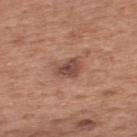Q: Was a biopsy performed?
A: no biopsy performed (imaged during a skin exam)
Q: What lighting was used for the tile?
A: white-light
Q: What did automated image analysis measure?
A: a mean CIELAB color near L≈48 a*≈22 b*≈26, roughly 11 lightness units darker than nearby skin, and a normalized lesion–skin contrast near 8; border irregularity of about 2 on a 0–10 scale, a within-lesion color-variation index near 3/10, and peripheral color asymmetry of about 1
Q: How large is the lesion?
A: about 3 mm
Q: Who is the patient?
A: male, aged approximately 55
Q: What kind of image is this?
A: total-body-photography crop, ~15 mm field of view
Q: Lesion location?
A: the upper back